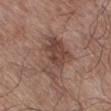biopsy status = total-body-photography surveillance lesion; no biopsy.